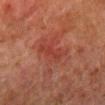image = ~15 mm crop, total-body skin-cancer survey | subject = male, in their 80s | image-analysis metrics = an area of roughly 6.5 mm², an eccentricity of roughly 0.9, and a symmetry-axis asymmetry near 0.35; a border-irregularity rating of about 4.5/10, a color-variation rating of about 2.5/10, and radial color variation of about 1 | site = the right lower leg | size = about 4.5 mm | tile lighting = cross-polarized illumination.